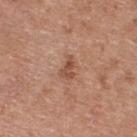<lesion>
  <biopsy_status>not biopsied; imaged during a skin examination</biopsy_status>
  <patient>
    <sex>female</sex>
    <age_approx>40</age_approx>
  </patient>
  <site>upper back</site>
  <image>
    <source>total-body photography crop</source>
    <field_of_view_mm>15</field_of_view_mm>
  </image>
  <automated_metrics>
    <cielab_L>51</cielab_L>
    <cielab_a>23</cielab_a>
    <cielab_b>30</cielab_b>
    <vs_skin_darker_L>10.0</vs_skin_darker_L>
    <vs_skin_contrast_norm>7.0</vs_skin_contrast_norm>
    <color_variation_0_10>2.0</color_variation_0_10>
    <peripheral_color_asymmetry>0.5</peripheral_color_asymmetry>
    <nevus_likeness_0_100>0</nevus_likeness_0_100>
  </automated_metrics>
  <lighting>white-light</lighting>
</lesion>